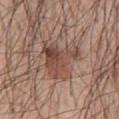Q: Is there a histopathology result?
A: imaged on a skin check; not biopsied
Q: How was this image acquired?
A: ~15 mm crop, total-body skin-cancer survey
Q: Lesion location?
A: the front of the torso
Q: Automated lesion metrics?
A: an average lesion color of about L≈47 a*≈19 b*≈26 (CIELAB); radial color variation of about 3; a classifier nevus-likeness of about 45/100
Q: What are the patient's age and sex?
A: male, aged 53–57
Q: What is the lesion's diameter?
A: ~5 mm (longest diameter)